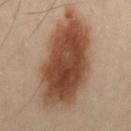illumination: cross-polarized | image source: total-body-photography crop, ~15 mm field of view | lesion diameter: about 11.5 mm | patient: male, approximately 50 years of age | location: the lower back.